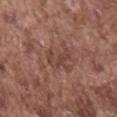notes: imaged on a skin check; not biopsied
patient: male, aged 73–77
tile lighting: white-light illumination
diameter: about 3.5 mm
imaging modality: total-body-photography crop, ~15 mm field of view
location: the mid back